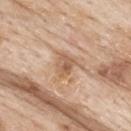Part of a total-body skin-imaging series; this lesion was reviewed on a skin check and was not flagged for biopsy. The lesion is located on the upper back. The tile uses white-light illumination. Approximately 3 mm at its widest. Automated image analysis of the tile measured an area of roughly 4.5 mm², a shape eccentricity near 0.65, and a symmetry-axis asymmetry near 0.35. The software also gave an average lesion color of about L≈58 a*≈19 b*≈32 (CIELAB) and a normalized lesion–skin contrast near 6.5. It also reported an automated nevus-likeness rating near 0 out of 100 and lesion-presence confidence of about 100/100. A male subject aged approximately 85 or older. This image is a 15 mm lesion crop taken from a total-body photograph.